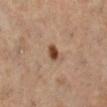Impression: The lesion was tiled from a total-body skin photograph and was not biopsied. Clinical summary: Automated tile analysis of the lesion measured a footprint of about 3.5 mm², an outline eccentricity of about 0.7 (0 = round, 1 = elongated), and a shape-asymmetry score of about 0.3 (0 = symmetric). And it measured a border-irregularity index near 2/10, internal color variation of about 3 on a 0–10 scale, and radial color variation of about 1. Located on the left lower leg. A female subject aged 53–57. A 15 mm close-up extracted from a 3D total-body photography capture. The tile uses cross-polarized illumination. Measured at roughly 2.5 mm in maximum diameter.